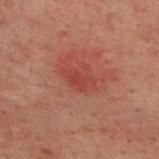{
  "biopsy_status": "not biopsied; imaged during a skin examination",
  "automated_metrics": {
    "cielab_L": 35,
    "cielab_a": 26,
    "cielab_b": 24,
    "vs_skin_darker_L": 5.0,
    "vs_skin_contrast_norm": 5.0,
    "border_irregularity_0_10": 3.5,
    "peripheral_color_asymmetry": 0.5
  },
  "lighting": "cross-polarized",
  "image": {
    "source": "total-body photography crop",
    "field_of_view_mm": 15
  },
  "patient": {
    "sex": "male",
    "age_approx": 40
  },
  "lesion_size": {
    "long_diameter_mm_approx": 3.5
  },
  "site": "upper back"
}